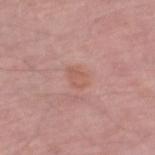{
  "lighting": "white-light",
  "lesion_size": {
    "long_diameter_mm_approx": 3.0
  },
  "image": {
    "source": "total-body photography crop",
    "field_of_view_mm": 15
  },
  "site": "left forearm",
  "patient": {
    "sex": "male",
    "age_approx": 65
  }
}